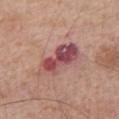Notes:
• workup: catalogued during a skin exam; not biopsied
• diameter: about 5.5 mm
• patient: male, aged approximately 65
• tile lighting: white-light illumination
• anatomic site: the left upper arm
• image source: ~15 mm tile from a whole-body skin photo